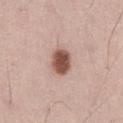Impression: Recorded during total-body skin imaging; not selected for excision or biopsy. Context: Approximately 3 mm at its widest. A male subject, roughly 45 years of age. On the leg. Cropped from a total-body skin-imaging series; the visible field is about 15 mm.